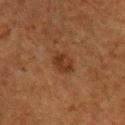The lesion was tiled from a total-body skin photograph and was not biopsied.
Longest diameter approximately 3 mm.
Imaged with cross-polarized lighting.
The lesion is located on the right upper arm.
Cropped from a total-body skin-imaging series; the visible field is about 15 mm.
The subject is a male in their 50s.
The lesion-visualizer software estimated an area of roughly 5.5 mm², an eccentricity of roughly 0.65, and two-axis asymmetry of about 0.2. The analysis additionally found a border-irregularity index near 2/10, a color-variation rating of about 2.5/10, and a peripheral color-asymmetry measure near 1.